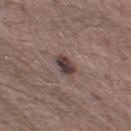<case>
  <biopsy_status>not biopsied; imaged during a skin examination</biopsy_status>
  <lighting>white-light</lighting>
  <automated_metrics>
    <cielab_L>39</cielab_L>
    <cielab_a>14</cielab_a>
    <cielab_b>17</cielab_b>
    <vs_skin_darker_L>13.0</vs_skin_darker_L>
    <vs_skin_contrast_norm>11.0</vs_skin_contrast_norm>
    <color_variation_0_10>4.5</color_variation_0_10>
    <peripheral_color_asymmetry>1.5</peripheral_color_asymmetry>
    <lesion_detection_confidence_0_100>100</lesion_detection_confidence_0_100>
  </automated_metrics>
  <site>leg</site>
  <lesion_size>
    <long_diameter_mm_approx>3.0</long_diameter_mm_approx>
  </lesion_size>
  <image>
    <source>total-body photography crop</source>
    <field_of_view_mm>15</field_of_view_mm>
  </image>
  <patient>
    <sex>male</sex>
    <age_approx>25</age_approx>
  </patient>
</case>